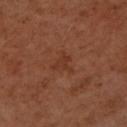Imaged during a routine full-body skin examination; the lesion was not biopsied and no histopathology is available. Cropped from a whole-body photographic skin survey; the tile spans about 15 mm. A female subject, roughly 50 years of age. Approximately 2.5 mm at its widest. From the arm. An algorithmic analysis of the crop reported border irregularity of about 5.5 on a 0–10 scale and a peripheral color-asymmetry measure near 0.5. The analysis additionally found a classifier nevus-likeness of about 0/100 and lesion-presence confidence of about 100/100.